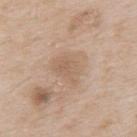Impression: The lesion was tiled from a total-body skin photograph and was not biopsied. Acquisition and patient details: Cropped from a total-body skin-imaging series; the visible field is about 15 mm. Captured under white-light illumination. The total-body-photography lesion software estimated an average lesion color of about L≈61 a*≈15 b*≈30 (CIELAB), a lesion–skin lightness drop of about 7, and a normalized border contrast of about 5. And it measured a border-irregularity rating of about 3.5/10, a color-variation rating of about 2.5/10, and peripheral color asymmetry of about 1. A male subject, aged 63–67. Longest diameter approximately 4 mm. On the upper back.